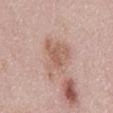No biopsy was performed on this lesion — it was imaged during a full skin examination and was not determined to be concerning.
The lesion's longest dimension is about 6 mm.
Cropped from a total-body skin-imaging series; the visible field is about 15 mm.
Located on the mid back.
A female patient aged 28 to 32.
Imaged with white-light lighting.